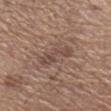Case summary:
- biopsy status: catalogued during a skin exam; not biopsied
- subject: male, about 65 years old
- anatomic site: the left forearm
- image source: ~15 mm tile from a whole-body skin photo